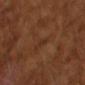| field | value |
|---|---|
| notes | imaged on a skin check; not biopsied |
| image source | total-body-photography crop, ~15 mm field of view |
| image-analysis metrics | a lesion area of about 2 mm², an eccentricity of roughly 0.95, and a symmetry-axis asymmetry near 0.45; border irregularity of about 4.5 on a 0–10 scale, a within-lesion color-variation index near 0/10, and radial color variation of about 0 |
| lighting | cross-polarized illumination |
| subject | male, in their mid- to late 60s |